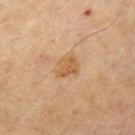A region of skin cropped from a whole-body photographic capture, roughly 15 mm wide. The lesion is on the left upper arm. A male patient aged approximately 70.